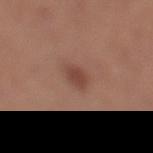The lesion was photographed on a routine skin check and not biopsied; there is no pathology result. This is a white-light tile. A female patient, approximately 40 years of age. A 15 mm crop from a total-body photograph taken for skin-cancer surveillance. On the left lower leg.